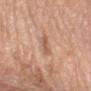This lesion was catalogued during total-body skin photography and was not selected for biopsy. The lesion is located on the right forearm. Imaged with cross-polarized lighting. The lesion-visualizer software estimated an average lesion color of about L≈55 a*≈20 b*≈30 (CIELAB), about 9 CIELAB-L* units darker than the surrounding skin, and a lesion-to-skin contrast of about 6 (normalized; higher = more distinct). The analysis additionally found an automated nevus-likeness rating near 0 out of 100. A male subject, in their 70s. About 3.5 mm across. A 15 mm close-up extracted from a 3D total-body photography capture.